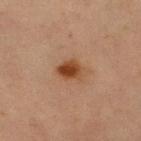follow-up=no biopsy performed (imaged during a skin exam) | image=15 mm crop, total-body photography | site=the right lower leg | subject=female, aged 68 to 72.